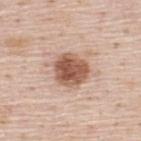biopsy status: imaged on a skin check; not biopsied
site: the upper back
acquisition: ~15 mm tile from a whole-body skin photo
patient: male, aged approximately 45
diameter: ~4 mm (longest diameter)